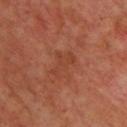Q: Was this lesion biopsied?
A: total-body-photography surveillance lesion; no biopsy
Q: What kind of image is this?
A: total-body-photography crop, ~15 mm field of view
Q: Where on the body is the lesion?
A: the chest
Q: What lighting was used for the tile?
A: cross-polarized
Q: What are the patient's age and sex?
A: male, aged 63 to 67